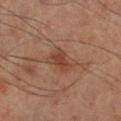subject = male, aged 48 to 52 | acquisition = ~15 mm crop, total-body skin-cancer survey | anatomic site = the right lower leg | size = ≈3 mm.